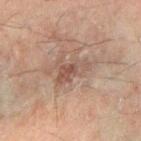Captured during whole-body skin photography for melanoma surveillance; the lesion was not biopsied. A close-up tile cropped from a whole-body skin photograph, about 15 mm across. A male patient approximately 45 years of age. From the right forearm.